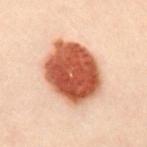Part of a total-body skin-imaging series; this lesion was reviewed on a skin check and was not flagged for biopsy.
The lesion is on the chest.
A 15 mm close-up extracted from a 3D total-body photography capture.
A female subject, aged 28 to 32.
The lesion's longest dimension is about 7 mm.